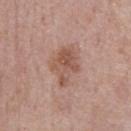The lesion was photographed on a routine skin check and not biopsied; there is no pathology result. Cropped from a total-body skin-imaging series; the visible field is about 15 mm. The lesion is located on the front of the torso. A male patient aged 73–77.